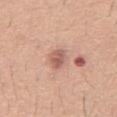follow-up — total-body-photography surveillance lesion; no biopsy | site — the abdomen | subject — male, aged 58–62 | acquisition — 15 mm crop, total-body photography | illumination — white-light | lesion size — ≈3 mm | image-analysis metrics — roughly 11 lightness units darker than nearby skin and a normalized border contrast of about 7.5.